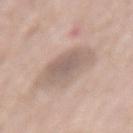  biopsy_status: not biopsied; imaged during a skin examination
  patient:
    sex: female
    age_approx: 75
  lighting: white-light
  automated_metrics:
    color_variation_0_10: 3.0
    peripheral_color_asymmetry: 1.0
  site: mid back
  image:
    source: total-body photography crop
    field_of_view_mm: 15